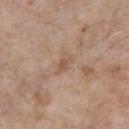follow-up = total-body-photography surveillance lesion; no biopsy